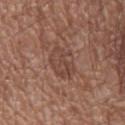Imaged during a routine full-body skin examination; the lesion was not biopsied and no histopathology is available. About 4 mm across. A region of skin cropped from a whole-body photographic capture, roughly 15 mm wide. A male subject approximately 55 years of age. From the leg. This is a white-light tile. An algorithmic analysis of the crop reported a footprint of about 9.5 mm², an outline eccentricity of about 0.75 (0 = round, 1 = elongated), and a shape-asymmetry score of about 0.25 (0 = symmetric). The analysis additionally found a mean CIELAB color near L≈44 a*≈19 b*≈25, roughly 7 lightness units darker than nearby skin, and a normalized border contrast of about 5.5. It also reported a detector confidence of about 100 out of 100 that the crop contains a lesion.